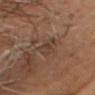Part of a total-body skin-imaging series; this lesion was reviewed on a skin check and was not flagged for biopsy. Located on the head or neck. Captured under cross-polarized illumination. A male subject, approximately 55 years of age. A 15 mm close-up tile from a total-body photography series done for melanoma screening.